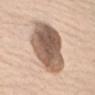Imaged with white-light lighting.
A region of skin cropped from a whole-body photographic capture, roughly 15 mm wide.
From the arm.
The recorded lesion diameter is about 6.5 mm.
A male patient, approximately 80 years of age.